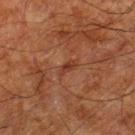This lesion was catalogued during total-body skin photography and was not selected for biopsy. Longest diameter approximately 2.5 mm. From the leg. Automated tile analysis of the lesion measured an area of roughly 2.5 mm², an outline eccentricity of about 0.9 (0 = round, 1 = elongated), and a shape-asymmetry score of about 0.35 (0 = symmetric). And it measured a lesion color around L≈29 a*≈21 b*≈25 in CIELAB, a lesion–skin lightness drop of about 6, and a normalized border contrast of about 6. The patient is a male about 80 years old. A region of skin cropped from a whole-body photographic capture, roughly 15 mm wide.